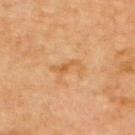Q: Was a biopsy performed?
A: imaged on a skin check; not biopsied
Q: Where on the body is the lesion?
A: the back
Q: How large is the lesion?
A: about 4 mm
Q: What is the imaging modality?
A: 15 mm crop, total-body photography
Q: Who is the patient?
A: in their mid- to late 60s
Q: What did automated image analysis measure?
A: an outline eccentricity of about 0.9 (0 = round, 1 = elongated) and a symmetry-axis asymmetry near 0.55; a border-irregularity index near 7/10, internal color variation of about 1 on a 0–10 scale, and peripheral color asymmetry of about 0; a nevus-likeness score of about 0/100
Q: How was the tile lit?
A: cross-polarized illumination